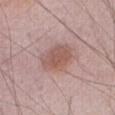– follow-up — total-body-photography surveillance lesion; no biopsy
– size — ~4.5 mm (longest diameter)
– location — the abdomen
– image — total-body-photography crop, ~15 mm field of view
– lighting — white-light illumination
– image-analysis metrics — a footprint of about 11 mm² and two-axis asymmetry of about 0.15; a classifier nevus-likeness of about 80/100
– patient — male, in their mid- to late 70s